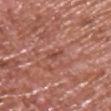{
  "biopsy_status": "not biopsied; imaged during a skin examination"
}